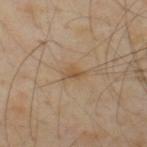{
  "biopsy_status": "not biopsied; imaged during a skin examination",
  "patient": {
    "sex": "male",
    "age_approx": 55
  },
  "site": "upper back",
  "image": {
    "source": "total-body photography crop",
    "field_of_view_mm": 15
  }
}